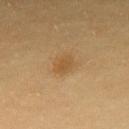Case summary:
* workup — no biopsy performed (imaged during a skin exam)
* acquisition — 15 mm crop, total-body photography
* automated metrics — a footprint of about 5 mm² and a shape eccentricity near 0.6
* body site — the back
* subject — female, aged 28–32
* size — about 3 mm